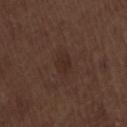Cropped from a total-body skin-imaging series; the visible field is about 15 mm. This is a white-light tile. Longest diameter approximately 3 mm. The subject is a male aged around 70. Located on the lower back.